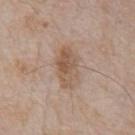Recorded during total-body skin imaging; not selected for excision or biopsy. Approximately 5 mm at its widest. A 15 mm crop from a total-body photograph taken for skin-cancer surveillance. A male patient in their 80s. The lesion-visualizer software estimated a footprint of about 9.5 mm², a shape eccentricity near 0.85, and two-axis asymmetry of about 0.15. The analysis additionally found roughly 9 lightness units darker than nearby skin and a normalized lesion–skin contrast near 7. On the chest. Imaged with white-light lighting.